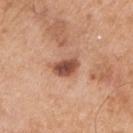No biopsy was performed on this lesion — it was imaged during a full skin examination and was not determined to be concerning.
From the arm.
The subject is a male in their mid-50s.
The total-body-photography lesion software estimated an area of roughly 6 mm² and a shape eccentricity near 0.75.
Cropped from a whole-body photographic skin survey; the tile spans about 15 mm.
The lesion's longest dimension is about 3 mm.
This is a white-light tile.